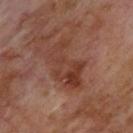Case summary:
• subject: male, aged 68 to 72
• diameter: ≈6 mm
• acquisition: ~15 mm crop, total-body skin-cancer survey
• lighting: cross-polarized
• anatomic site: the upper back